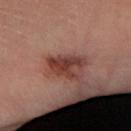Acquisition and patient details:
About 4 mm across. The subject is a male aged around 40. A close-up tile cropped from a whole-body skin photograph, about 15 mm across. On the arm.